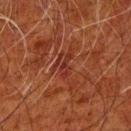<tbp_lesion>
<automated_metrics>
  <shape_asymmetry>0.45</shape_asymmetry>
</automated_metrics>
<patient>
  <sex>male</sex>
  <age_approx>80</age_approx>
</patient>
<image>
  <source>total-body photography crop</source>
  <field_of_view_mm>15</field_of_view_mm>
</image>
<site>arm</site>
<lesion_size>
  <long_diameter_mm_approx>3.0</long_diameter_mm_approx>
</lesion_size>
</tbp_lesion>